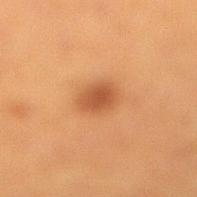A lesion tile, about 15 mm wide, cut from a 3D total-body photograph.
Measured at roughly 3 mm in maximum diameter.
The lesion is located on the right lower leg.
Automated image analysis of the tile measured an average lesion color of about L≈44 a*≈23 b*≈33 (CIELAB). The analysis additionally found a detector confidence of about 100 out of 100 that the crop contains a lesion.
A female patient, roughly 40 years of age.